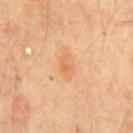Clinical impression:
No biopsy was performed on this lesion — it was imaged during a full skin examination and was not determined to be concerning.
Background:
Captured under cross-polarized illumination. The lesion's longest dimension is about 3.5 mm. The lesion is on the chest. A 15 mm close-up extracted from a 3D total-body photography capture. The patient is a male in their mid-60s.